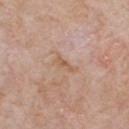Recorded during total-body skin imaging; not selected for excision or biopsy.
A 15 mm crop from a total-body photograph taken for skin-cancer surveillance.
A male subject aged 58 to 62.
On the front of the torso.